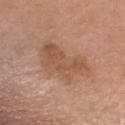The lesion was tiled from a total-body skin photograph and was not biopsied.
A female patient, in their 40s.
A close-up tile cropped from a whole-body skin photograph, about 15 mm across.
The lesion is located on the head or neck.